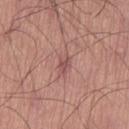Q: Is there a histopathology result?
A: no biopsy performed (imaged during a skin exam)
Q: Lesion location?
A: the left thigh
Q: Automated lesion metrics?
A: a lesion area of about 3 mm² and two-axis asymmetry of about 0.25; radial color variation of about 0.5
Q: How was the tile lit?
A: white-light
Q: What kind of image is this?
A: ~15 mm crop, total-body skin-cancer survey
Q: Who is the patient?
A: male, in their mid-50s